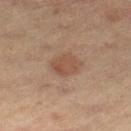No biopsy was performed on this lesion — it was imaged during a full skin examination and was not determined to be concerning.
A male subject, about 65 years old.
Captured under cross-polarized illumination.
A 15 mm crop from a total-body photograph taken for skin-cancer surveillance.
The recorded lesion diameter is about 3.5 mm.
Located on the left thigh.
The total-body-photography lesion software estimated a footprint of about 6.5 mm². The software also gave an average lesion color of about L≈44 a*≈18 b*≈26 (CIELAB), a lesion–skin lightness drop of about 7, and a normalized lesion–skin contrast near 6.